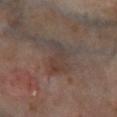* biopsy status: imaged on a skin check; not biopsied
* lesion size: ~4.5 mm (longest diameter)
* acquisition: ~15 mm tile from a whole-body skin photo
* automated metrics: a mean CIELAB color near L≈37 a*≈13 b*≈19, a lesion–skin lightness drop of about 6, and a normalized lesion–skin contrast near 5.5; a nevus-likeness score of about 0/100 and lesion-presence confidence of about 85/100
* subject: male, aged approximately 70
* tile lighting: cross-polarized
* anatomic site: the left lower leg